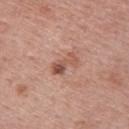Q: Was a biopsy performed?
A: total-body-photography surveillance lesion; no biopsy
Q: Where on the body is the lesion?
A: the upper back
Q: What are the patient's age and sex?
A: female, in their mid-50s
Q: Illumination type?
A: white-light illumination
Q: How was this image acquired?
A: ~15 mm crop, total-body skin-cancer survey
Q: What did automated image analysis measure?
A: a lesion area of about 5 mm², an eccentricity of roughly 0.9, and a shape-asymmetry score of about 0.35 (0 = symmetric); a lesion–skin lightness drop of about 10; a border-irregularity rating of about 4.5/10 and a color-variation rating of about 5/10